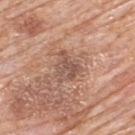Case summary:
* workup: total-body-photography surveillance lesion; no biopsy
* image source: total-body-photography crop, ~15 mm field of view
* anatomic site: the upper back
* automated metrics: a mean CIELAB color near L≈53 a*≈20 b*≈27, roughly 9 lightness units darker than nearby skin, and a lesion-to-skin contrast of about 6.5 (normalized; higher = more distinct); border irregularity of about 5.5 on a 0–10 scale, internal color variation of about 3.5 on a 0–10 scale, and peripheral color asymmetry of about 1.5; a detector confidence of about 100 out of 100 that the crop contains a lesion
* subject: male, aged 78 to 82
* lesion diameter: ~3.5 mm (longest diameter)
* tile lighting: white-light